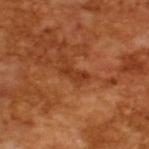{
  "biopsy_status": "not biopsied; imaged during a skin examination",
  "patient": {
    "sex": "male",
    "age_approx": 65
  },
  "image": {
    "source": "total-body photography crop",
    "field_of_view_mm": 15
  }
}